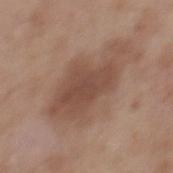The lesion was tiled from a total-body skin photograph and was not biopsied. The subject is a male about 55 years old. Approximately 8.5 mm at its widest. On the mid back. A 15 mm close-up tile from a total-body photography series done for melanoma screening.